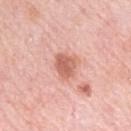Impression:
Recorded during total-body skin imaging; not selected for excision or biopsy.
Acquisition and patient details:
This is a white-light tile. The lesion is on the left upper arm. A female subject aged 63 to 67. A region of skin cropped from a whole-body photographic capture, roughly 15 mm wide. The recorded lesion diameter is about 3 mm.